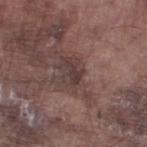Impression:
Captured during whole-body skin photography for melanoma surveillance; the lesion was not biopsied.
Context:
On the left thigh. The recorded lesion diameter is about 3 mm. A 15 mm close-up tile from a total-body photography series done for melanoma screening. Imaged with white-light lighting. A male patient, roughly 75 years of age.